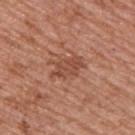Imaged during a routine full-body skin examination; the lesion was not biopsied and no histopathology is available. A male patient aged approximately 55. A roughly 15 mm field-of-view crop from a total-body skin photograph. Measured at roughly 4 mm in maximum diameter. An algorithmic analysis of the crop reported a shape eccentricity near 0.8 and a symmetry-axis asymmetry near 0.35. The analysis additionally found a mean CIELAB color near L≈49 a*≈24 b*≈31, a lesion–skin lightness drop of about 8, and a normalized lesion–skin contrast near 6.5. The software also gave a border-irregularity index near 4/10, a within-lesion color-variation index near 2.5/10, and radial color variation of about 1. The analysis additionally found a nevus-likeness score of about 5/100 and a detector confidence of about 100 out of 100 that the crop contains a lesion. The tile uses white-light illumination. On the upper back.